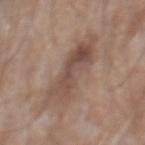<lesion>
<biopsy_status>not biopsied; imaged during a skin examination</biopsy_status>
<site>mid back</site>
<image>
  <source>total-body photography crop</source>
  <field_of_view_mm>15</field_of_view_mm>
</image>
<lesion_size>
  <long_diameter_mm_approx>8.0</long_diameter_mm_approx>
</lesion_size>
<lighting>white-light</lighting>
<patient>
  <sex>male</sex>
  <age_approx>60</age_approx>
</patient>
</lesion>